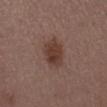Imaged during a routine full-body skin examination; the lesion was not biopsied and no histopathology is available. The patient is a male about 50 years old. A 15 mm close-up extracted from a 3D total-body photography capture. From the mid back. This is a white-light tile.